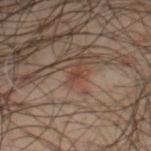Captured during whole-body skin photography for melanoma surveillance; the lesion was not biopsied. Captured under cross-polarized illumination. The total-body-photography lesion software estimated a lesion area of about 1 mm², an outline eccentricity of about 0.7 (0 = round, 1 = elongated), and two-axis asymmetry of about 0.4. The analysis additionally found a mean CIELAB color near L≈40 a*≈21 b*≈26, about 6 CIELAB-L* units darker than the surrounding skin, and a normalized lesion–skin contrast near 6. And it measured border irregularity of about 3 on a 0–10 scale and a peripheral color-asymmetry measure near 0. The software also gave an automated nevus-likeness rating near 10 out of 100 and a detector confidence of about 100 out of 100 that the crop contains a lesion. A lesion tile, about 15 mm wide, cut from a 3D total-body photograph. A male subject aged 58 to 62. The lesion is on the chest.